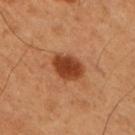Assessment:
Part of a total-body skin-imaging series; this lesion was reviewed on a skin check and was not flagged for biopsy.
Image and clinical context:
On the right upper arm. A male patient, aged around 50. Imaged with cross-polarized lighting. The lesion's longest dimension is about 4 mm. A region of skin cropped from a whole-body photographic capture, roughly 15 mm wide.